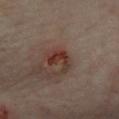The lesion was photographed on a routine skin check and not biopsied; there is no pathology result.